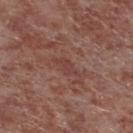Assessment: The lesion was photographed on a routine skin check and not biopsied; there is no pathology result. Image and clinical context: A close-up tile cropped from a whole-body skin photograph, about 15 mm across. The lesion is on the leg. Approximately 2.5 mm at its widest. A male subject, roughly 55 years of age.